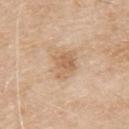Approximately 3.5 mm at its widest. A male patient aged approximately 80. A 15 mm close-up tile from a total-body photography series done for melanoma screening. The lesion is on the back.